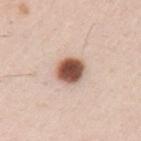  biopsy_status: not biopsied; imaged during a skin examination
  patient:
    sex: male
    age_approx: 35
  image:
    source: total-body photography crop
    field_of_view_mm: 15
  lesion_size:
    long_diameter_mm_approx: 3.5
  automated_metrics:
    area_mm2_approx: 8.0
    eccentricity: 0.55
    shape_asymmetry: 0.2
    border_irregularity_0_10: 1.5
    color_variation_0_10: 5.0
    peripheral_color_asymmetry: 1.0
  site: left upper arm